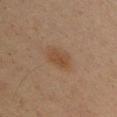Recorded during total-body skin imaging; not selected for excision or biopsy.
Imaged with cross-polarized lighting.
A male patient, about 50 years old.
Located on the head or neck.
A 15 mm close-up extracted from a 3D total-body photography capture.
Longest diameter approximately 3.5 mm.